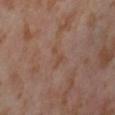{
  "biopsy_status": "not biopsied; imaged during a skin examination",
  "lighting": "cross-polarized",
  "patient": {
    "sex": "female",
    "age_approx": 55
  },
  "automated_metrics": {
    "area_mm2_approx": 3.5,
    "shape_asymmetry": 0.65,
    "cielab_L": 48,
    "cielab_a": 18,
    "cielab_b": 28,
    "vs_skin_darker_L": 4.0,
    "vs_skin_contrast_norm": 5.0
  },
  "image": {
    "source": "total-body photography crop",
    "field_of_view_mm": 15
  },
  "site": "left thigh"
}